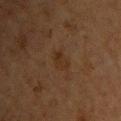Recorded during total-body skin imaging; not selected for excision or biopsy. The lesion is located on the left upper arm. A lesion tile, about 15 mm wide, cut from a 3D total-body photograph. This is a cross-polarized tile. The total-body-photography lesion software estimated an area of roughly 3.5 mm², an outline eccentricity of about 0.8 (0 = round, 1 = elongated), and two-axis asymmetry of about 0.35. The analysis additionally found a color-variation rating of about 1.5/10 and peripheral color asymmetry of about 0.5. And it measured a classifier nevus-likeness of about 5/100 and lesion-presence confidence of about 100/100. The patient is a female approximately 55 years of age.